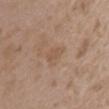Clinical summary:
The patient is a female approximately 35 years of age. This image is a 15 mm lesion crop taken from a total-body photograph. From the left upper arm.